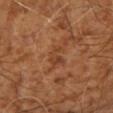{
  "biopsy_status": "not biopsied; imaged during a skin examination",
  "site": "right upper arm",
  "image": {
    "source": "total-body photography crop",
    "field_of_view_mm": 15
  },
  "patient": {
    "age_approx": 65
  }
}